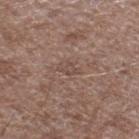biopsy status = imaged on a skin check; not biopsied | patient = male, roughly 70 years of age | body site = the right lower leg | acquisition = 15 mm crop, total-body photography.